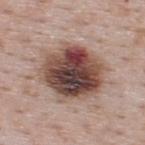workup = catalogued during a skin exam; not biopsied
body site = the upper back
image = ~15 mm tile from a whole-body skin photo
size = about 7 mm
tile lighting = white-light illumination
patient = male, aged approximately 50